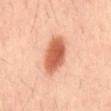biopsy_status: not biopsied; imaged during a skin examination
automated_metrics:
  area_mm2_approx: 12.0
  eccentricity: 0.85
  shape_asymmetry: 0.1
  color_variation_0_10: 4.0
  peripheral_color_asymmetry: 1.0
patient:
  sex: male
  age_approx: 40
image:
  source: total-body photography crop
  field_of_view_mm: 15
lighting: cross-polarized
lesion_size:
  long_diameter_mm_approx: 5.0
site: back